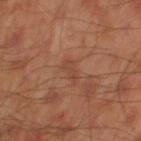subject: male, approximately 45 years of age
lesion size: ≈3 mm
illumination: cross-polarized illumination
site: the left thigh
acquisition: ~15 mm tile from a whole-body skin photo